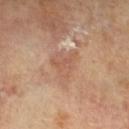follow-up = no biopsy performed (imaged during a skin exam) | diameter = ≈5 mm | lighting = cross-polarized illumination | site = the leg | subject = female, in their mid-70s | imaging modality = 15 mm crop, total-body photography.